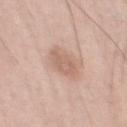The lesion was tiled from a total-body skin photograph and was not biopsied.
A female patient about 60 years old.
The lesion's longest dimension is about 3 mm.
A close-up tile cropped from a whole-body skin photograph, about 15 mm across.
The lesion is on the left thigh.
Captured under white-light illumination.